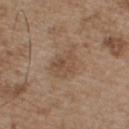follow-up — catalogued during a skin exam; not biopsied | subject — male, about 55 years old | image — ~15 mm crop, total-body skin-cancer survey | tile lighting — white-light | location — the chest.